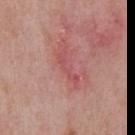Impression:
This lesion was catalogued during total-body skin photography and was not selected for biopsy.
Acquisition and patient details:
A close-up tile cropped from a whole-body skin photograph, about 15 mm across. Longest diameter approximately 4 mm. The tile uses white-light illumination. A male patient, aged approximately 50. Located on the front of the torso.